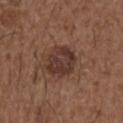Recorded during total-body skin imaging; not selected for excision or biopsy. Longest diameter approximately 4 mm. A close-up tile cropped from a whole-body skin photograph, about 15 mm across. Imaged with white-light lighting. Located on the right upper arm. The patient is a male aged approximately 50.